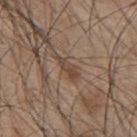Notes:
• follow-up — no biopsy performed (imaged during a skin exam)
• automated lesion analysis — an area of roughly 4 mm², an outline eccentricity of about 0.95 (0 = round, 1 = elongated), and two-axis asymmetry of about 0.4; a classifier nevus-likeness of about 5/100 and a detector confidence of about 80 out of 100 that the crop contains a lesion
• tile lighting — white-light
• body site — the back
• image — ~15 mm crop, total-body skin-cancer survey
• lesion diameter — ≈3.5 mm
• patient — male, aged 43–47